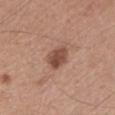Recorded during total-body skin imaging; not selected for excision or biopsy. A male patient aged 53 to 57. A roughly 15 mm field-of-view crop from a total-body skin photograph. The lesion is located on the mid back.